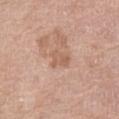biopsy status = imaged on a skin check; not biopsied | patient = female, in their mid- to late 60s | anatomic site = the left thigh | image = ~15 mm crop, total-body skin-cancer survey.